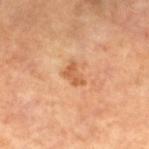Imaged during a routine full-body skin examination; the lesion was not biopsied and no histopathology is available.
A 15 mm crop from a total-body photograph taken for skin-cancer surveillance.
The lesion is on the leg.
This is a cross-polarized tile.
A female patient aged 63 to 67.